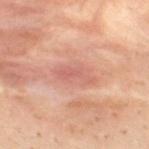biopsy status=total-body-photography surveillance lesion; no biopsy
subject=male, aged around 30
imaging modality=~15 mm crop, total-body skin-cancer survey
location=the mid back